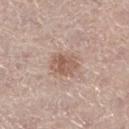image-analysis metrics: a lesion area of about 7 mm², a shape eccentricity near 0.7, and a symmetry-axis asymmetry near 0.2; an average lesion color of about L≈57 a*≈18 b*≈27 (CIELAB), about 10 CIELAB-L* units darker than the surrounding skin, and a normalized lesion–skin contrast near 7; a detector confidence of about 100 out of 100 that the crop contains a lesion
tile lighting: white-light illumination
size: ~3.5 mm (longest diameter)
acquisition: 15 mm crop, total-body photography
anatomic site: the right lower leg
patient: female, in their mid- to late 60s